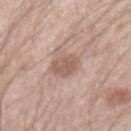• follow-up — no biopsy performed (imaged during a skin exam)
• lesion size — ≈3 mm
• image source — ~15 mm tile from a whole-body skin photo
• lighting — white-light
• automated lesion analysis — a lesion color around L≈57 a*≈19 b*≈25 in CIELAB and a normalized border contrast of about 6.5
• body site — the left forearm
• patient — male, roughly 45 years of age